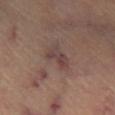The lesion was tiled from a total-body skin photograph and was not biopsied.
From the left leg.
Automated image analysis of the tile measured a footprint of about 5 mm² and an outline eccentricity of about 0.75 (0 = round, 1 = elongated). And it measured a lesion color around L≈41 a*≈18 b*≈19 in CIELAB, about 8 CIELAB-L* units darker than the surrounding skin, and a normalized lesion–skin contrast near 7. And it measured a border-irregularity index near 4.5/10, a within-lesion color-variation index near 4.5/10, and a peripheral color-asymmetry measure near 1.5. The analysis additionally found lesion-presence confidence of about 95/100.
A lesion tile, about 15 mm wide, cut from a 3D total-body photograph.
A female patient, about 60 years old.